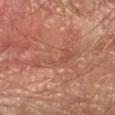diameter = ~6.5 mm (longest diameter)
body site = the arm
acquisition = 15 mm crop, total-body photography
automated metrics = a footprint of about 10 mm², a shape eccentricity near 0.95, and two-axis asymmetry of about 0.65; a border-irregularity index near 9.5/10, a within-lesion color-variation index near 2/10, and peripheral color asymmetry of about 0.5
tile lighting = cross-polarized illumination